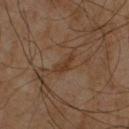Recorded during total-body skin imaging; not selected for excision or biopsy. A male subject, in their 60s. Imaged with cross-polarized lighting. The lesion-visualizer software estimated an area of roughly 3 mm². The analysis additionally found internal color variation of about 1 on a 0–10 scale and radial color variation of about 0. The lesion's longest dimension is about 3 mm. Cropped from a whole-body photographic skin survey; the tile spans about 15 mm. On the chest.